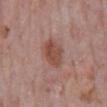Imaged during a routine full-body skin examination; the lesion was not biopsied and no histopathology is available.
Longest diameter approximately 5 mm.
Cropped from a whole-body photographic skin survey; the tile spans about 15 mm.
The patient is a male about 75 years old.
On the back.